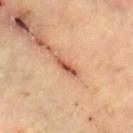Q: Is there a histopathology result?
A: total-body-photography surveillance lesion; no biopsy
Q: Who is the patient?
A: approximately 60 years of age
Q: What is the anatomic site?
A: the right lower leg
Q: How was this image acquired?
A: ~15 mm crop, total-body skin-cancer survey